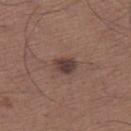biopsy status: no biopsy performed (imaged during a skin exam) | patient: male, in their mid-60s | imaging modality: ~15 mm crop, total-body skin-cancer survey | lesion diameter: ≈3 mm | illumination: white-light illumination | automated lesion analysis: an area of roughly 5.5 mm², an outline eccentricity of about 0.7 (0 = round, 1 = elongated), and a shape-asymmetry score of about 0.25 (0 = symmetric); a mean CIELAB color near L≈39 a*≈17 b*≈20, roughly 11 lightness units darker than nearby skin, and a normalized border contrast of about 9; internal color variation of about 4.5 on a 0–10 scale and radial color variation of about 1.5; an automated nevus-likeness rating near 80 out of 100 | anatomic site: the right thigh.